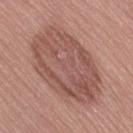| feature | finding |
|---|---|
| follow-up | no biopsy performed (imaged during a skin exam) |
| patient | female, aged around 65 |
| anatomic site | the left thigh |
| size | ~10.5 mm (longest diameter) |
| acquisition | ~15 mm tile from a whole-body skin photo |